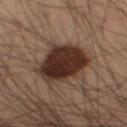Impression:
Recorded during total-body skin imaging; not selected for excision or biopsy.
Background:
A 15 mm crop from a total-body photograph taken for skin-cancer surveillance. Measured at roughly 7 mm in maximum diameter. The lesion is located on the right thigh. A male subject, approximately 55 years of age. Captured under cross-polarized illumination. The total-body-photography lesion software estimated an average lesion color of about L≈24 a*≈14 b*≈19 (CIELAB) and a lesion–skin lightness drop of about 13. The analysis additionally found internal color variation of about 3.5 on a 0–10 scale and a peripheral color-asymmetry measure near 1. The analysis additionally found an automated nevus-likeness rating near 90 out of 100 and lesion-presence confidence of about 100/100.